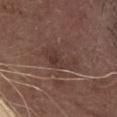This lesion was catalogued during total-body skin photography and was not selected for biopsy.
A 15 mm close-up tile from a total-body photography series done for melanoma screening.
The patient is a female in their 80s.
On the head or neck.
Longest diameter approximately 5 mm.
This is a white-light tile.